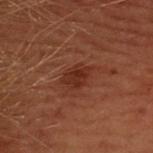Located on the upper back.
A region of skin cropped from a whole-body photographic capture, roughly 15 mm wide.
The subject is a female in their 50s.